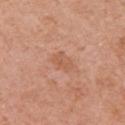The lesion was photographed on a routine skin check and not biopsied; there is no pathology result.
A female patient, aged approximately 60.
The lesion is on the left upper arm.
Automated tile analysis of the lesion measured a footprint of about 4.5 mm², an eccentricity of roughly 0.75, and a shape-asymmetry score of about 0.2 (0 = symmetric). It also reported a border-irregularity rating of about 2.5/10, a color-variation rating of about 1.5/10, and radial color variation of about 0.5. And it measured a classifier nevus-likeness of about 0/100 and a detector confidence of about 100 out of 100 that the crop contains a lesion.
Captured under white-light illumination.
Measured at roughly 3 mm in maximum diameter.
A region of skin cropped from a whole-body photographic capture, roughly 15 mm wide.